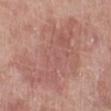No biopsy was performed on this lesion — it was imaged during a full skin examination and was not determined to be concerning. The total-body-photography lesion software estimated an average lesion color of about L≈57 a*≈23 b*≈25 (CIELAB), about 7 CIELAB-L* units darker than the surrounding skin, and a normalized border contrast of about 5. The analysis additionally found a within-lesion color-variation index near 3.5/10 and peripheral color asymmetry of about 1. It also reported a nevus-likeness score of about 0/100 and a detector confidence of about 100 out of 100 that the crop contains a lesion. The subject is a female in their 70s. This is a white-light tile. Located on the left lower leg. Cropped from a total-body skin-imaging series; the visible field is about 15 mm.